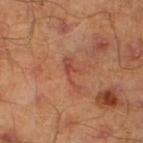  biopsy_status: not biopsied; imaged during a skin examination
  site: left lower leg
  lesion_size:
    long_diameter_mm_approx: 4.0
  automated_metrics:
    cielab_L: 47
    cielab_a: 27
    cielab_b: 31
    vs_skin_darker_L: 7.0
    border_irregularity_0_10: 9.5
    color_variation_0_10: 2.0
  image:
    source: total-body photography crop
    field_of_view_mm: 15
  lighting: cross-polarized
  patient:
    sex: male
    age_approx: 45